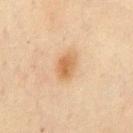biopsy status: catalogued during a skin exam; not biopsied | imaging modality: 15 mm crop, total-body photography | diameter: about 3.5 mm | tile lighting: cross-polarized illumination | subject: male, aged around 65 | anatomic site: the chest.